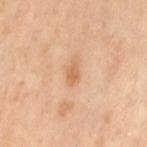Imaged during a routine full-body skin examination; the lesion was not biopsied and no histopathology is available. From the left thigh. A female patient in their mid- to late 50s. Automated image analysis of the tile measured a lesion area of about 3 mm² and an eccentricity of roughly 0.85. The software also gave a border-irregularity rating of about 3/10, internal color variation of about 1.5 on a 0–10 scale, and peripheral color asymmetry of about 0.5. The analysis additionally found a lesion-detection confidence of about 100/100. Cropped from a whole-body photographic skin survey; the tile spans about 15 mm. This is a cross-polarized tile.